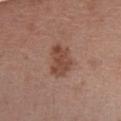No biopsy was performed on this lesion — it was imaged during a full skin examination and was not determined to be concerning. A 15 mm close-up tile from a total-body photography series done for melanoma screening. A female subject, aged around 40. The tile uses white-light illumination. Longest diameter approximately 4.5 mm. From the chest. The lesion-visualizer software estimated a mean CIELAB color near L≈46 a*≈21 b*≈28 and a lesion-to-skin contrast of about 7.5 (normalized; higher = more distinct). It also reported a border-irregularity index near 2.5/10, a color-variation rating of about 3/10, and radial color variation of about 1. And it measured an automated nevus-likeness rating near 25 out of 100.